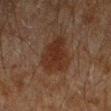Recorded during total-body skin imaging; not selected for excision or biopsy.
The lesion is located on the arm.
A male subject, aged around 45.
Cropped from a whole-body photographic skin survey; the tile spans about 15 mm.
The recorded lesion diameter is about 5 mm.
Imaged with cross-polarized lighting.